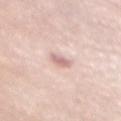Q: Was a biopsy performed?
A: imaged on a skin check; not biopsied
Q: Lesion location?
A: the chest
Q: What is the imaging modality?
A: ~15 mm tile from a whole-body skin photo
Q: What are the patient's age and sex?
A: female, about 50 years old
Q: Illumination type?
A: white-light
Q: What is the lesion's diameter?
A: about 2.5 mm